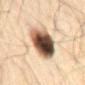Findings:
* acquisition · ~15 mm crop, total-body skin-cancer survey
* anatomic site · the mid back
* subject · male, aged 58 to 62
* pathology · a seborrheic keratosis